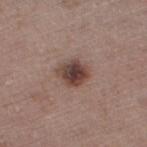A male subject, aged around 65. A region of skin cropped from a whole-body photographic capture, roughly 15 mm wide. The lesion is on the left thigh.